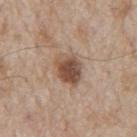<record>
<biopsy_status>not biopsied; imaged during a skin examination</biopsy_status>
<automated_metrics>
  <nevus_likeness_0_100>95</nevus_likeness_0_100>
  <lesion_detection_confidence_0_100>100</lesion_detection_confidence_0_100>
</automated_metrics>
<lesion_size>
  <long_diameter_mm_approx>4.0</long_diameter_mm_approx>
</lesion_size>
<patient>
  <sex>male</sex>
  <age_approx>65</age_approx>
</patient>
<site>back</site>
<lighting>white-light</lighting>
<image>
  <source>total-body photography crop</source>
  <field_of_view_mm>15</field_of_view_mm>
</image>
</record>